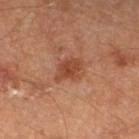<lesion>
  <biopsy_status>not biopsied; imaged during a skin examination</biopsy_status>
  <image>
    <source>total-body photography crop</source>
    <field_of_view_mm>15</field_of_view_mm>
  </image>
  <patient>
    <sex>male</sex>
    <age_approx>65</age_approx>
  </patient>
  <site>left lower leg</site>
</lesion>